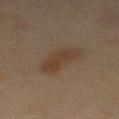| field | value |
|---|---|
| notes | no biopsy performed (imaged during a skin exam) |
| lighting | cross-polarized illumination |
| subject | male, aged 58–62 |
| diameter | ~5 mm (longest diameter) |
| location | the front of the torso |
| TBP lesion metrics | a within-lesion color-variation index near 3/10; an automated nevus-likeness rating near 35 out of 100 and lesion-presence confidence of about 100/100 |
| image | 15 mm crop, total-body photography |